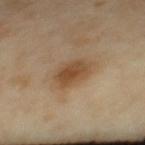No biopsy was performed on this lesion — it was imaged during a full skin examination and was not determined to be concerning. On the upper back. This is a cross-polarized tile. An algorithmic analysis of the crop reported a footprint of about 10 mm² and an eccentricity of roughly 0.8. The software also gave a lesion–skin lightness drop of about 10 and a normalized border contrast of about 8. It also reported a classifier nevus-likeness of about 95/100 and a detector confidence of about 100 out of 100 that the crop contains a lesion. A female patient roughly 45 years of age. A 15 mm close-up tile from a total-body photography series done for melanoma screening.